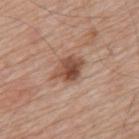A 15 mm close-up tile from a total-body photography series done for melanoma screening.
From the mid back.
The patient is a male aged approximately 80.
The total-body-photography lesion software estimated a lesion color around L≈49 a*≈21 b*≈29 in CIELAB, roughly 13 lightness units darker than nearby skin, and a normalized border contrast of about 9.5.
The tile uses white-light illumination.